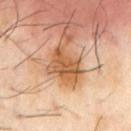Context:
The tile uses cross-polarized illumination. The recorded lesion diameter is about 4 mm. This image is a 15 mm lesion crop taken from a total-body photograph. The subject is a male roughly 65 years of age. The total-body-photography lesion software estimated an outline eccentricity of about 0.8 (0 = round, 1 = elongated) and a symmetry-axis asymmetry near 0.4. And it measured a mean CIELAB color near L≈54 a*≈23 b*≈37, a lesion–skin lightness drop of about 11, and a normalized lesion–skin contrast near 8.5. The software also gave internal color variation of about 4 on a 0–10 scale and a peripheral color-asymmetry measure near 1.5. Located on the front of the torso.